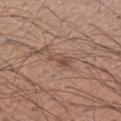Recorded during total-body skin imaging; not selected for excision or biopsy. Imaged with white-light lighting. A roughly 15 mm field-of-view crop from a total-body skin photograph. A male subject, aged around 55.